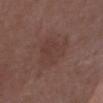notes: catalogued during a skin exam; not biopsied | subject: male, aged 73 to 77 | size: ≈5.5 mm | automated metrics: an area of roughly 13 mm², a shape eccentricity near 0.8, and a shape-asymmetry score of about 0.35 (0 = symmetric); an average lesion color of about L≈38 a*≈18 b*≈22 (CIELAB), a lesion–skin lightness drop of about 5, and a normalized lesion–skin contrast near 4.5 | lighting: white-light illumination | image source: total-body-photography crop, ~15 mm field of view | anatomic site: the chest.